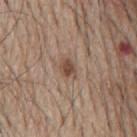| feature | finding |
|---|---|
| notes | imaged on a skin check; not biopsied |
| tile lighting | white-light illumination |
| imaging modality | total-body-photography crop, ~15 mm field of view |
| patient | male, aged around 65 |
| location | the back |